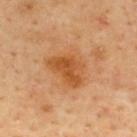Q: Was a biopsy performed?
A: no biopsy performed (imaged during a skin exam)